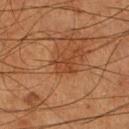A 15 mm close-up extracted from a 3D total-body photography capture. A male patient, roughly 55 years of age. The lesion's longest dimension is about 2.5 mm. Imaged with cross-polarized lighting. From the right lower leg.